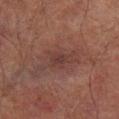biopsy status = imaged on a skin check; not biopsied | image-analysis metrics = an automated nevus-likeness rating near 0 out of 100 | site = the right lower leg | illumination = cross-polarized | image = 15 mm crop, total-body photography | subject = male, aged approximately 75.